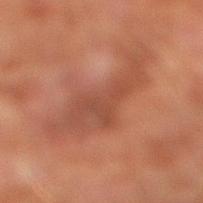The lesion was photographed on a routine skin check and not biopsied; there is no pathology result.
Located on the right lower leg.
Automated tile analysis of the lesion measured a classifier nevus-likeness of about 0/100 and lesion-presence confidence of about 100/100.
The tile uses cross-polarized illumination.
A male patient aged 73–77.
A close-up tile cropped from a whole-body skin photograph, about 15 mm across.